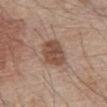Q: How large is the lesion?
A: ≈4.5 mm
Q: Illumination type?
A: white-light
Q: What kind of image is this?
A: total-body-photography crop, ~15 mm field of view
Q: What is the anatomic site?
A: the abdomen
Q: Who is the patient?
A: male, in their 80s